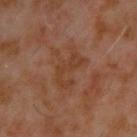Recorded during total-body skin imaging; not selected for excision or biopsy.
A male subject, approximately 60 years of age.
Located on the upper back.
A lesion tile, about 15 mm wide, cut from a 3D total-body photograph.
Approximately 5 mm at its widest.
An algorithmic analysis of the crop reported a color-variation rating of about 2/10. It also reported an automated nevus-likeness rating near 0 out of 100.